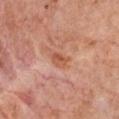Captured during whole-body skin photography for melanoma surveillance; the lesion was not biopsied. A 15 mm crop from a total-body photograph taken for skin-cancer surveillance. The lesion-visualizer software estimated an average lesion color of about L≈56 a*≈27 b*≈34 (CIELAB) and roughly 8 lightness units darker than nearby skin. The analysis additionally found a border-irregularity rating of about 2.5/10, a within-lesion color-variation index near 4/10, and radial color variation of about 1.5. And it measured an automated nevus-likeness rating near 0 out of 100 and a detector confidence of about 100 out of 100 that the crop contains a lesion. A female patient in their 60s. The lesion is located on the chest. This is a white-light tile. Approximately 2.5 mm at its widest.